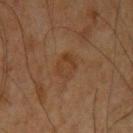| feature | finding |
|---|---|
| follow-up | total-body-photography surveillance lesion; no biopsy |
| acquisition | total-body-photography crop, ~15 mm field of view |
| anatomic site | the right upper arm |
| patient | male, aged 58–62 |